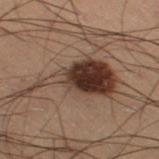Impression:
The lesion was photographed on a routine skin check and not biopsied; there is no pathology result.
Background:
This is a cross-polarized tile. From the right thigh. A male subject in their mid- to late 50s. The recorded lesion diameter is about 9.5 mm. A lesion tile, about 15 mm wide, cut from a 3D total-body photograph.